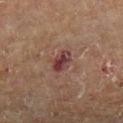Impression:
This lesion was catalogued during total-body skin photography and was not selected for biopsy.
Background:
Captured under cross-polarized illumination. Located on the left lower leg. A male subject, aged 83 or over. A close-up tile cropped from a whole-body skin photograph, about 15 mm across. Automated image analysis of the tile measured roughly 11 lightness units darker than nearby skin and a normalized lesion–skin contrast near 10.5. And it measured border irregularity of about 1.5 on a 0–10 scale, a within-lesion color-variation index near 6/10, and radial color variation of about 2.